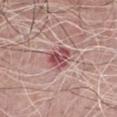Located on the chest.
The subject is a male in their mid-60s.
A region of skin cropped from a whole-body photographic capture, roughly 15 mm wide.